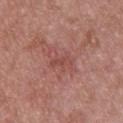follow-up — catalogued during a skin exam; not biopsied | diameter — ≈3 mm | acquisition — ~15 mm tile from a whole-body skin photo | image-analysis metrics — an average lesion color of about L≈48 a*≈26 b*≈25 (CIELAB), a lesion–skin lightness drop of about 7, and a lesion-to-skin contrast of about 5 (normalized; higher = more distinct) | illumination — white-light illumination | anatomic site — the upper back | patient — male, aged 38 to 42.